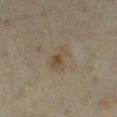patient = female, in their mid-30s; lesion size = ≈3.5 mm; imaging modality = ~15 mm tile from a whole-body skin photo; tile lighting = cross-polarized illumination; location = the left thigh.